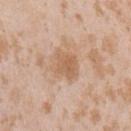{
  "biopsy_status": "not biopsied; imaged during a skin examination",
  "site": "arm",
  "patient": {
    "sex": "female",
    "age_approx": 25
  },
  "image": {
    "source": "total-body photography crop",
    "field_of_view_mm": 15
  }
}